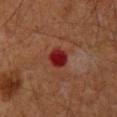Case summary:
– notes · imaged on a skin check; not biopsied